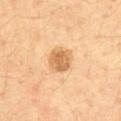<case>
  <biopsy_status>not biopsied; imaged during a skin examination</biopsy_status>
  <patient>
    <sex>male</sex>
    <age_approx>60</age_approx>
  </patient>
  <site>abdomen</site>
  <lesion_size>
    <long_diameter_mm_approx>3.0</long_diameter_mm_approx>
  </lesion_size>
  <lighting>cross-polarized</lighting>
  <image>
    <source>total-body photography crop</source>
    <field_of_view_mm>15</field_of_view_mm>
  </image>
</case>